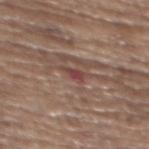- workup: total-body-photography surveillance lesion; no biopsy
- body site: the upper back
- imaging modality: total-body-photography crop, ~15 mm field of view
- subject: female, aged 63 to 67